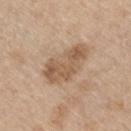Context: Automated tile analysis of the lesion measured a lesion area of about 14 mm² and two-axis asymmetry of about 0.3. The software also gave a mean CIELAB color near L≈56 a*≈17 b*≈32 and a lesion–skin lightness drop of about 11. It also reported a border-irregularity rating of about 4.5/10, internal color variation of about 3.5 on a 0–10 scale, and peripheral color asymmetry of about 1. A 15 mm crop from a total-body photograph taken for skin-cancer surveillance. The recorded lesion diameter is about 6.5 mm. Located on the right thigh. The tile uses white-light illumination. A male subject, aged approximately 70.